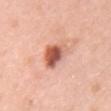Findings:
* follow-up — total-body-photography surveillance lesion; no biopsy